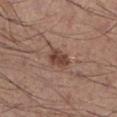Assessment:
This lesion was catalogued during total-body skin photography and was not selected for biopsy.
Acquisition and patient details:
Measured at roughly 3 mm in maximum diameter. A 15 mm crop from a total-body photograph taken for skin-cancer surveillance. A male patient, in their mid- to late 30s. The lesion is located on the left lower leg.